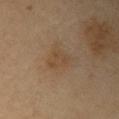A 15 mm crop from a total-body photograph taken for skin-cancer surveillance. The patient is a male in their mid-80s or older. Located on the arm.